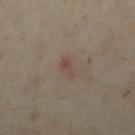| feature | finding |
|---|---|
| notes | no biopsy performed (imaged during a skin exam) |
| subject | female, aged 33 to 37 |
| lesion size | ≈3 mm |
| image | ~15 mm crop, total-body skin-cancer survey |
| body site | the right lower leg |
| automated metrics | an area of roughly 3 mm², a shape eccentricity near 0.85, and two-axis asymmetry of about 0.45; an average lesion color of about L≈43 a*≈15 b*≈22 (CIELAB), roughly 6 lightness units darker than nearby skin, and a normalized lesion–skin contrast near 5; a border-irregularity rating of about 4.5/10 and a within-lesion color-variation index near 1/10; an automated nevus-likeness rating near 0 out of 100 |